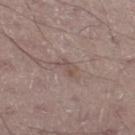  biopsy_status: not biopsied; imaged during a skin examination
  site: left thigh
  patient:
    sex: male
    age_approx: 60
  image:
    source: total-body photography crop
    field_of_view_mm: 15
  lesion_size:
    long_diameter_mm_approx: 2.5